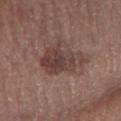Part of a total-body skin-imaging series; this lesion was reviewed on a skin check and was not flagged for biopsy. Approximately 5 mm at its widest. A region of skin cropped from a whole-body photographic capture, roughly 15 mm wide. On the right forearm. Automated image analysis of the tile measured a footprint of about 15 mm² and a shape-asymmetry score of about 0.4 (0 = symmetric). The software also gave border irregularity of about 5 on a 0–10 scale, internal color variation of about 4.5 on a 0–10 scale, and a peripheral color-asymmetry measure near 1.5. The subject is a female approximately 65 years of age.